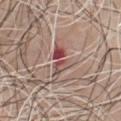Findings:
• biopsy status — no biopsy performed (imaged during a skin exam)
• imaging modality — total-body-photography crop, ~15 mm field of view
• location — the front of the torso
• patient — male, aged 63 to 67